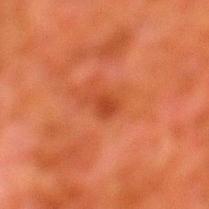Assessment:
Recorded during total-body skin imaging; not selected for excision or biopsy.
Context:
This image is a 15 mm lesion crop taken from a total-body photograph. A male patient, approximately 80 years of age. An algorithmic analysis of the crop reported a lesion area of about 3.5 mm², a shape eccentricity near 0.8, and a symmetry-axis asymmetry near 0.35. It also reported an average lesion color of about L≈37 a*≈30 b*≈35 (CIELAB), a lesion–skin lightness drop of about 7, and a lesion-to-skin contrast of about 6 (normalized; higher = more distinct). From the left lower leg.